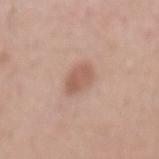biopsy status: no biopsy performed (imaged during a skin exam)
subject: male, in their mid-50s
image: ~15 mm crop, total-body skin-cancer survey
anatomic site: the mid back